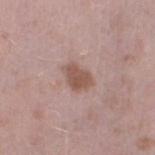The lesion was photographed on a routine skin check and not biopsied; there is no pathology result.